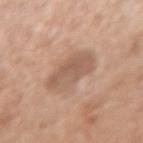The lesion was tiled from a total-body skin photograph and was not biopsied. An algorithmic analysis of the crop reported an area of roughly 13 mm² and an eccentricity of roughly 0.75. The software also gave a lesion color around L≈57 a*≈18 b*≈29 in CIELAB, a lesion–skin lightness drop of about 10, and a lesion-to-skin contrast of about 6.5 (normalized; higher = more distinct). It also reported a border-irregularity rating of about 2/10, internal color variation of about 3 on a 0–10 scale, and radial color variation of about 1. And it measured a nevus-likeness score of about 0/100 and a detector confidence of about 100 out of 100 that the crop contains a lesion. Imaged with white-light lighting. The patient is a female aged approximately 50. A 15 mm close-up tile from a total-body photography series done for melanoma screening. On the left forearm. The lesion's longest dimension is about 5 mm.